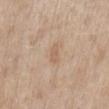Notes:
* automated lesion analysis · a lesion area of about 3 mm², a shape eccentricity near 0.85, and two-axis asymmetry of about 0.3; a lesion color around L≈61 a*≈16 b*≈31 in CIELAB, a lesion–skin lightness drop of about 7, and a normalized border contrast of about 5; border irregularity of about 2.5 on a 0–10 scale and peripheral color asymmetry of about 0; a nevus-likeness score of about 0/100 and a detector confidence of about 100 out of 100 that the crop contains a lesion
* imaging modality · ~15 mm tile from a whole-body skin photo
* anatomic site · the mid back
* diameter · about 2.5 mm
* patient · female, aged 58–62